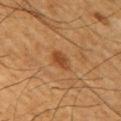Automated tile analysis of the lesion measured an area of roughly 3.5 mm², an outline eccentricity of about 0.75 (0 = round, 1 = elongated), and a shape-asymmetry score of about 0.2 (0 = symmetric). The software also gave an average lesion color of about L≈41 a*≈23 b*≈35 (CIELAB), about 9 CIELAB-L* units darker than the surrounding skin, and a lesion-to-skin contrast of about 7.5 (normalized; higher = more distinct). It also reported an automated nevus-likeness rating near 80 out of 100. The tile uses cross-polarized illumination. Approximately 2.5 mm at its widest. The lesion is located on the arm. A close-up tile cropped from a whole-body skin photograph, about 15 mm across. A male subject roughly 60 years of age.